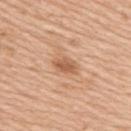{
  "biopsy_status": "not biopsied; imaged during a skin examination"
}